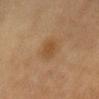Impression: Part of a total-body skin-imaging series; this lesion was reviewed on a skin check and was not flagged for biopsy. Image and clinical context: The lesion is on the abdomen. Measured at roughly 3 mm in maximum diameter. A male subject, aged 53 to 57. Imaged with cross-polarized lighting. A region of skin cropped from a whole-body photographic capture, roughly 15 mm wide. Automated tile analysis of the lesion measured a nevus-likeness score of about 45/100 and a lesion-detection confidence of about 100/100.